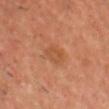{
  "biopsy_status": "not biopsied; imaged during a skin examination",
  "automated_metrics": {
    "area_mm2_approx": 5.0,
    "eccentricity": 0.7,
    "shape_asymmetry": 0.2,
    "nevus_likeness_0_100": 0,
    "lesion_detection_confidence_0_100": 100
  },
  "lighting": "cross-polarized",
  "patient": {
    "sex": "male",
    "age_approx": 40
  },
  "site": "head or neck",
  "lesion_size": {
    "long_diameter_mm_approx": 3.0
  },
  "image": {
    "source": "total-body photography crop",
    "field_of_view_mm": 15
  }
}